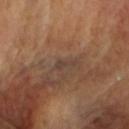The lesion was photographed on a routine skin check and not biopsied; there is no pathology result. From the head or neck. A female patient in their mid- to late 50s. Approximately 2.5 mm at its widest. Captured under cross-polarized illumination. The total-body-photography lesion software estimated a footprint of about 2.5 mm², an eccentricity of roughly 0.9, and two-axis asymmetry of about 0.5. It also reported a lesion color around L≈41 a*≈16 b*≈24 in CIELAB, roughly 5 lightness units darker than nearby skin, and a normalized lesion–skin contrast near 6. And it measured a border-irregularity rating of about 5/10, internal color variation of about 0 on a 0–10 scale, and radial color variation of about 0. A 15 mm close-up extracted from a 3D total-body photography capture.